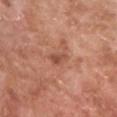Located on the leg.
A male subject approximately 80 years of age.
Automated image analysis of the tile measured a lesion area of about 3 mm², a shape eccentricity near 0.85, and a symmetry-axis asymmetry near 0.3. The software also gave a lesion color around L≈51 a*≈25 b*≈31 in CIELAB, about 9 CIELAB-L* units darker than the surrounding skin, and a lesion-to-skin contrast of about 6.5 (normalized; higher = more distinct). The analysis additionally found a nevus-likeness score of about 0/100 and a lesion-detection confidence of about 100/100.
Cropped from a total-body skin-imaging series; the visible field is about 15 mm.
About 2.5 mm across.